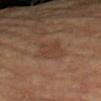Recorded during total-body skin imaging; not selected for excision or biopsy.
The tile uses cross-polarized illumination.
The recorded lesion diameter is about 4 mm.
A female patient, aged 63 to 67.
A 15 mm crop from a total-body photograph taken for skin-cancer surveillance.
An algorithmic analysis of the crop reported an average lesion color of about L≈39 a*≈18 b*≈27 (CIELAB), a lesion–skin lightness drop of about 6, and a normalized border contrast of about 5.
The lesion is on the left forearm.